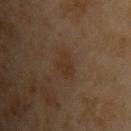{
  "biopsy_status": "not biopsied; imaged during a skin examination",
  "lighting": "cross-polarized",
  "automated_metrics": {
    "area_mm2_approx": 5.0,
    "eccentricity": 0.35,
    "shape_asymmetry": 0.2,
    "nevus_likeness_0_100": 10,
    "lesion_detection_confidence_0_100": 100
  },
  "image": {
    "source": "total-body photography crop",
    "field_of_view_mm": 15
  },
  "lesion_size": {
    "long_diameter_mm_approx": 2.5
  },
  "site": "chest",
  "patient": {
    "sex": "male",
    "age_approx": 55
  }
}